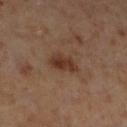biopsy_status: not biopsied; imaged during a skin examination
image:
  source: total-body photography crop
  field_of_view_mm: 15
patient:
  sex: male
  age_approx: 60
lesion_size:
  long_diameter_mm_approx: 3.5
lighting: cross-polarized
site: left lower leg